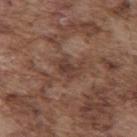Context: Imaged with white-light lighting. Longest diameter approximately 3.5 mm. Automated image analysis of the tile measured a border-irregularity rating of about 5/10 and a within-lesion color-variation index near 3.5/10. It also reported an automated nevus-likeness rating near 0 out of 100 and a lesion-detection confidence of about 60/100. From the mid back. A male patient about 75 years old. A region of skin cropped from a whole-body photographic capture, roughly 15 mm wide.